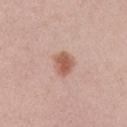  lesion_size:
    long_diameter_mm_approx: 3.0
  image:
    source: total-body photography crop
    field_of_view_mm: 15
  site: arm
  lighting: white-light
  patient:
    sex: female
    age_approx: 40
  automated_metrics:
    area_mm2_approx: 6.0
    eccentricity: 0.65
    shape_asymmetry: 0.25
    cielab_L: 58
    cielab_a: 22
    cielab_b: 29
    vs_skin_darker_L: 12.0
    vs_skin_contrast_norm: 8.5
    border_irregularity_0_10: 2.5
    peripheral_color_asymmetry: 0.5